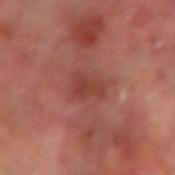Part of a total-body skin-imaging series; this lesion was reviewed on a skin check and was not flagged for biopsy. Located on the arm. Automated tile analysis of the lesion measured a border-irregularity rating of about 4/10, a within-lesion color-variation index near 3.5/10, and radial color variation of about 1. Imaged with cross-polarized lighting. About 3 mm across. Cropped from a whole-body photographic skin survey; the tile spans about 15 mm. A male subject approximately 65 years of age.